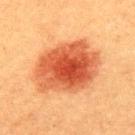This lesion was catalogued during total-body skin photography and was not selected for biopsy.
Cropped from a whole-body photographic skin survey; the tile spans about 15 mm.
The subject is a male approximately 40 years of age.
Captured under cross-polarized illumination.
The lesion is located on the upper back.